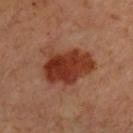notes — total-body-photography surveillance lesion; no biopsy.